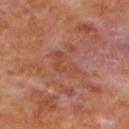biopsy status — catalogued during a skin exam; not biopsied | image — total-body-photography crop, ~15 mm field of view | automated lesion analysis — an automated nevus-likeness rating near 0 out of 100 | location — the upper back | tile lighting — cross-polarized | subject — female, approximately 50 years of age.